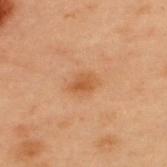| feature | finding |
|---|---|
| workup | imaged on a skin check; not biopsied |
| imaging modality | total-body-photography crop, ~15 mm field of view |
| site | the upper back |
| subject | male, aged 53 to 57 |
| lesion diameter | ≈3 mm |